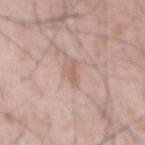Q: Is there a histopathology result?
A: imaged on a skin check; not biopsied
Q: What are the patient's age and sex?
A: male, approximately 55 years of age
Q: What is the imaging modality?
A: ~15 mm crop, total-body skin-cancer survey
Q: Lesion size?
A: ≈3 mm
Q: What did automated image analysis measure?
A: roughly 8 lightness units darker than nearby skin and a normalized lesion–skin contrast near 5; a lesion-detection confidence of about 95/100
Q: What is the anatomic site?
A: the mid back
Q: Illumination type?
A: white-light illumination